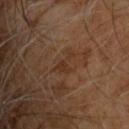biopsy status: catalogued during a skin exam; not biopsied
patient: male, about 60 years old
lesion size: ≈3 mm
lighting: cross-polarized illumination
anatomic site: the upper back
imaging modality: ~15 mm crop, total-body skin-cancer survey